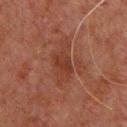Clinical impression:
Part of a total-body skin-imaging series; this lesion was reviewed on a skin check and was not flagged for biopsy.
Acquisition and patient details:
Located on the chest. A male subject, aged 58 to 62. The recorded lesion diameter is about 3 mm. Cropped from a whole-body photographic skin survey; the tile spans about 15 mm. Captured under cross-polarized illumination.